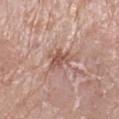Imaged during a routine full-body skin examination; the lesion was not biopsied and no histopathology is available.
The lesion-visualizer software estimated an area of roughly 5 mm², an eccentricity of roughly 0.8, and a shape-asymmetry score of about 0.4 (0 = symmetric). The software also gave a border-irregularity index near 5/10, a within-lesion color-variation index near 2/10, and a peripheral color-asymmetry measure near 0.5. It also reported a nevus-likeness score of about 0/100.
The lesion is on the right lower leg.
A female subject approximately 55 years of age.
Cropped from a total-body skin-imaging series; the visible field is about 15 mm.
The tile uses white-light illumination.
Approximately 3.5 mm at its widest.